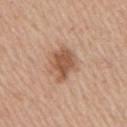notes: total-body-photography surveillance lesion; no biopsy | diameter: ~4.5 mm (longest diameter) | anatomic site: the right upper arm | image source: ~15 mm tile from a whole-body skin photo | illumination: white-light illumination | TBP lesion metrics: an area of roughly 10 mm², a shape eccentricity near 0.7, and two-axis asymmetry of about 0.35; border irregularity of about 4 on a 0–10 scale and peripheral color asymmetry of about 1; a nevus-likeness score of about 50/100 and lesion-presence confidence of about 100/100 | subject: male, in their mid-70s.